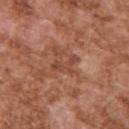Impression: Part of a total-body skin-imaging series; this lesion was reviewed on a skin check and was not flagged for biopsy.